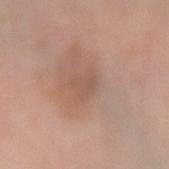Q: What is the anatomic site?
A: the right forearm
Q: Patient demographics?
A: female, roughly 55 years of age
Q: Lesion size?
A: ≈3 mm
Q: What did automated image analysis measure?
A: a nevus-likeness score of about 20/100 and lesion-presence confidence of about 95/100
Q: How was the tile lit?
A: white-light
Q: What is the imaging modality?
A: ~15 mm crop, total-body skin-cancer survey